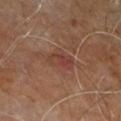Q: Was a biopsy performed?
A: catalogued during a skin exam; not biopsied
Q: What are the patient's age and sex?
A: male, roughly 60 years of age
Q: What lighting was used for the tile?
A: cross-polarized illumination
Q: What is the imaging modality?
A: ~15 mm crop, total-body skin-cancer survey
Q: What did automated image analysis measure?
A: an area of roughly 7 mm² and two-axis asymmetry of about 0.2; an average lesion color of about L≈39 a*≈21 b*≈25 (CIELAB) and roughly 6 lightness units darker than nearby skin; a border-irregularity rating of about 2/10 and radial color variation of about 1.5
Q: How large is the lesion?
A: ~3.5 mm (longest diameter)
Q: Lesion location?
A: the right leg